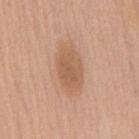Recorded during total-body skin imaging; not selected for excision or biopsy. This is a white-light tile. The patient is a female approximately 60 years of age. A 15 mm close-up tile from a total-body photography series done for melanoma screening. The lesion is on the mid back.